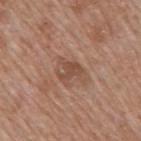The lesion was tiled from a total-body skin photograph and was not biopsied.
Imaged with white-light lighting.
Cropped from a total-body skin-imaging series; the visible field is about 15 mm.
Automated tile analysis of the lesion measured a lesion color around L≈48 a*≈20 b*≈29 in CIELAB and a normalized lesion–skin contrast near 6.5.
The subject is a male aged approximately 60.
From the mid back.